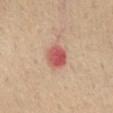Recorded during total-body skin imaging; not selected for excision or biopsy. Automated tile analysis of the lesion measured an area of roughly 7 mm², a shape eccentricity near 0.4, and a symmetry-axis asymmetry near 0.15. It also reported an average lesion color of about L≈57 a*≈31 b*≈27 (CIELAB), roughly 14 lightness units darker than nearby skin, and a normalized border contrast of about 9. The software also gave border irregularity of about 1.5 on a 0–10 scale and internal color variation of about 5.5 on a 0–10 scale. It also reported an automated nevus-likeness rating near 0 out of 100 and a detector confidence of about 100 out of 100 that the crop contains a lesion. A close-up tile cropped from a whole-body skin photograph, about 15 mm across. A female patient, aged around 60. The lesion's longest dimension is about 3 mm. The lesion is on the chest.